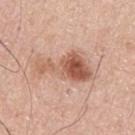Captured during whole-body skin photography for melanoma surveillance; the lesion was not biopsied.
The total-body-photography lesion software estimated border irregularity of about 7 on a 0–10 scale and internal color variation of about 6 on a 0–10 scale.
The tile uses white-light illumination.
From the back.
The subject is a male aged approximately 30.
A 15 mm close-up extracted from a 3D total-body photography capture.